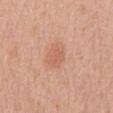{"biopsy_status": "not biopsied; imaged during a skin examination", "site": "abdomen", "patient": {"sex": "male", "age_approx": 70}, "image": {"source": "total-body photography crop", "field_of_view_mm": 15}, "lesion_size": {"long_diameter_mm_approx": 3.0}, "lighting": "white-light"}